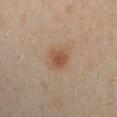Part of a total-body skin-imaging series; this lesion was reviewed on a skin check and was not flagged for biopsy.
Approximately 3.5 mm at its widest.
Automated tile analysis of the lesion measured a lesion area of about 6.5 mm² and a symmetry-axis asymmetry near 0.25. And it measured an automated nevus-likeness rating near 80 out of 100 and a lesion-detection confidence of about 100/100.
The lesion is located on the arm.
A region of skin cropped from a whole-body photographic capture, roughly 15 mm wide.
The patient is a female aged around 40.
Imaged with cross-polarized lighting.